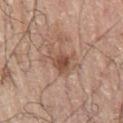Recorded during total-body skin imaging; not selected for excision or biopsy.
A lesion tile, about 15 mm wide, cut from a 3D total-body photograph.
From the left arm.
The patient is a male in their 70s.
The tile uses white-light illumination.
The recorded lesion diameter is about 4 mm.